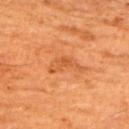Notes:
* workup · no biopsy performed (imaged during a skin exam)
* lighting · cross-polarized
* image-analysis metrics · a lesion area of about 5.5 mm², a shape eccentricity near 0.9, and two-axis asymmetry of about 0.5; a within-lesion color-variation index near 2.5/10 and a peripheral color-asymmetry measure near 0.5; a nevus-likeness score of about 0/100 and lesion-presence confidence of about 100/100
* subject · male, in their mid- to late 60s
* body site · the back
* lesion size · ≈4.5 mm
* image · ~15 mm crop, total-body skin-cancer survey